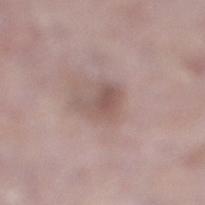Q: What is the anatomic site?
A: the right lower leg
Q: What are the patient's age and sex?
A: male, in their mid- to late 70s
Q: How was this image acquired?
A: total-body-photography crop, ~15 mm field of view
Q: Lesion size?
A: ~3.5 mm (longest diameter)